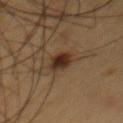Findings:
- follow-up — total-body-photography surveillance lesion; no biopsy
- image source — ~15 mm crop, total-body skin-cancer survey
- TBP lesion metrics — an area of roughly 6.5 mm², an eccentricity of roughly 0.9, and a symmetry-axis asymmetry near 0.2; a mean CIELAB color near L≈31 a*≈16 b*≈26, about 12 CIELAB-L* units darker than the surrounding skin, and a lesion-to-skin contrast of about 11 (normalized; higher = more distinct); border irregularity of about 2.5 on a 0–10 scale, a color-variation rating of about 4.5/10, and radial color variation of about 1
- subject — male, about 60 years old
- illumination — cross-polarized
- anatomic site — the chest